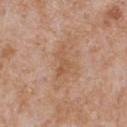Q: Is there a histopathology result?
A: imaged on a skin check; not biopsied
Q: What kind of image is this?
A: ~15 mm crop, total-body skin-cancer survey
Q: Patient demographics?
A: male, aged around 65
Q: Lesion location?
A: the chest